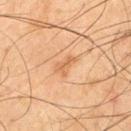Notes:
• biopsy status · total-body-photography surveillance lesion; no biopsy
• lesion diameter · ~3 mm (longest diameter)
• image source · total-body-photography crop, ~15 mm field of view
• tile lighting · cross-polarized illumination
• patient · male, aged 43 to 47
• body site · the back